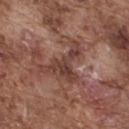Notes:
• follow-up · no biopsy performed (imaged during a skin exam)
• imaging modality · total-body-photography crop, ~15 mm field of view
• body site · the upper back
• patient · male, approximately 75 years of age